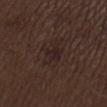Case summary:
- follow-up — catalogued during a skin exam; not biopsied
- lighting — white-light illumination
- location — the mid back
- subject — male, aged approximately 70
- imaging modality — total-body-photography crop, ~15 mm field of view
- size — about 4.5 mm
- automated lesion analysis — an average lesion color of about L≈22 a*≈13 b*≈17 (CIELAB) and about 5 CIELAB-L* units darker than the surrounding skin; a detector confidence of about 100 out of 100 that the crop contains a lesion